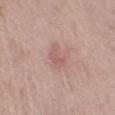Impression: The lesion was photographed on a routine skin check and not biopsied; there is no pathology result. Image and clinical context: A close-up tile cropped from a whole-body skin photograph, about 15 mm across. A male patient, in their 60s. Captured under white-light illumination. Approximately 3 mm at its widest. From the right lower leg. Automated tile analysis of the lesion measured a mean CIELAB color near L≈59 a*≈21 b*≈23 and a normalized lesion–skin contrast near 4.5. The analysis additionally found a border-irregularity index near 3.5/10, a within-lesion color-variation index near 3.5/10, and radial color variation of about 1.5. It also reported an automated nevus-likeness rating near 0 out of 100 and a detector confidence of about 100 out of 100 that the crop contains a lesion.